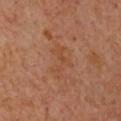{"biopsy_status": "not biopsied; imaged during a skin examination", "site": "chest", "lighting": "cross-polarized", "lesion_size": {"long_diameter_mm_approx": 4.5}, "patient": {"sex": "female", "age_approx": 40}, "image": {"source": "total-body photography crop", "field_of_view_mm": 15}, "automated_metrics": {"eccentricity": 0.9, "shape_asymmetry": 0.65, "border_irregularity_0_10": 8.5, "color_variation_0_10": 0.5, "peripheral_color_asymmetry": 0.0, "nevus_likeness_0_100": 0, "lesion_detection_confidence_0_100": 100}}